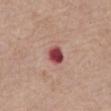Part of a total-body skin-imaging series; this lesion was reviewed on a skin check and was not flagged for biopsy. The lesion-visualizer software estimated an area of roughly 5 mm², an eccentricity of roughly 0.6, and two-axis asymmetry of about 0.2. It also reported a lesion–skin lightness drop of about 18 and a lesion-to-skin contrast of about 12.5 (normalized; higher = more distinct). Cropped from a whole-body photographic skin survey; the tile spans about 15 mm. The tile uses white-light illumination. On the front of the torso. Approximately 2.5 mm at its widest. A male subject aged approximately 65.